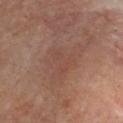biopsy status = total-body-photography surveillance lesion; no biopsy | lesion size = about 6.5 mm | image = ~15 mm tile from a whole-body skin photo | patient = male, aged approximately 65 | body site = the head or neck.